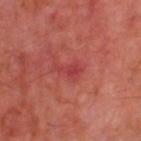follow-up: imaged on a skin check; not biopsied
automated lesion analysis: a color-variation rating of about 1.5/10; a detector confidence of about 100 out of 100 that the crop contains a lesion
diameter: ≈3 mm
body site: the left thigh
patient: male, aged 68–72
illumination: cross-polarized
image: ~15 mm tile from a whole-body skin photo